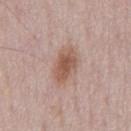{
  "biopsy_status": "not biopsied; imaged during a skin examination",
  "lesion_size": {
    "long_diameter_mm_approx": 4.5
  },
  "image": {
    "source": "total-body photography crop",
    "field_of_view_mm": 15
  },
  "lighting": "white-light",
  "automated_metrics": {
    "cielab_L": 55,
    "cielab_a": 20,
    "cielab_b": 27,
    "vs_skin_darker_L": 11.0,
    "border_irregularity_0_10": 2.0,
    "color_variation_0_10": 3.5,
    "peripheral_color_asymmetry": 1.0
  },
  "patient": {
    "sex": "male",
    "age_approx": 50
  },
  "site": "chest"
}